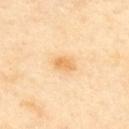Part of a total-body skin-imaging series; this lesion was reviewed on a skin check and was not flagged for biopsy.
The lesion is on the upper back.
Automated image analysis of the tile measured an area of roughly 4 mm², an eccentricity of roughly 0.75, and a symmetry-axis asymmetry near 0.15. And it measured a border-irregularity index near 1.5/10, internal color variation of about 3 on a 0–10 scale, and peripheral color asymmetry of about 1. The analysis additionally found a lesion-detection confidence of about 100/100.
A close-up tile cropped from a whole-body skin photograph, about 15 mm across.
Captured under cross-polarized illumination.
A female subject, aged 38–42.